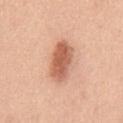Captured under white-light illumination. A male subject, about 50 years old. A roughly 15 mm field-of-view crop from a total-body skin photograph. From the front of the torso. An algorithmic analysis of the crop reported a lesion area of about 12 mm², an outline eccentricity of about 0.85 (0 = round, 1 = elongated), and a symmetry-axis asymmetry near 0.15. The analysis additionally found a lesion-detection confidence of about 100/100.